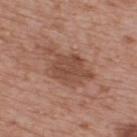{"biopsy_status": "not biopsied; imaged during a skin examination", "patient": {"sex": "male", "age_approx": 75}, "image": {"source": "total-body photography crop", "field_of_view_mm": 15}, "lesion_size": {"long_diameter_mm_approx": 5.5}, "site": "upper back", "lighting": "white-light"}